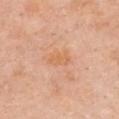{"biopsy_status": "not biopsied; imaged during a skin examination", "image": {"source": "total-body photography crop", "field_of_view_mm": 15}, "lesion_size": {"long_diameter_mm_approx": 3.0}, "patient": {"sex": "female", "age_approx": 45}, "site": "chest", "lighting": "white-light", "automated_metrics": {"cielab_L": 65, "cielab_a": 24, "cielab_b": 39, "vs_skin_darker_L": 6.0, "vs_skin_contrast_norm": 6.0, "border_irregularity_0_10": 3.5, "color_variation_0_10": 1.5, "peripheral_color_asymmetry": 0.5, "nevus_likeness_0_100": 0, "lesion_detection_confidence_0_100": 100}}